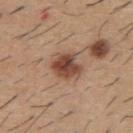Imaged during a routine full-body skin examination; the lesion was not biopsied and no histopathology is available. The lesion-visualizer software estimated an area of roughly 10 mm², an outline eccentricity of about 0.6 (0 = round, 1 = elongated), and a symmetry-axis asymmetry near 0.2. And it measured an average lesion color of about L≈47 a*≈21 b*≈28 (CIELAB) and a lesion–skin lightness drop of about 14. And it measured border irregularity of about 2.5 on a 0–10 scale, a color-variation rating of about 6/10, and peripheral color asymmetry of about 1.5. The analysis additionally found a nevus-likeness score of about 100/100 and a lesion-detection confidence of about 100/100. This image is a 15 mm lesion crop taken from a total-body photograph. The lesion is located on the upper back. The patient is a male aged 58 to 62. The tile uses white-light illumination.